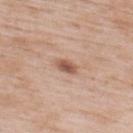Findings:
- illumination · white-light illumination
- image-analysis metrics · a mean CIELAB color near L≈55 a*≈20 b*≈29 and a normalized border contrast of about 8.5
- image · ~15 mm crop, total-body skin-cancer survey
- site · the upper back
- patient · male, aged 48 to 52